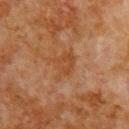Clinical impression: No biopsy was performed on this lesion — it was imaged during a full skin examination and was not determined to be concerning. Background: The lesion-visualizer software estimated a footprint of about 6 mm², an outline eccentricity of about 0.75 (0 = round, 1 = elongated), and a shape-asymmetry score of about 0.45 (0 = symmetric). The lesion is on the right upper arm. A 15 mm close-up extracted from a 3D total-body photography capture. Longest diameter approximately 4 mm. A male patient, roughly 80 years of age. Captured under cross-polarized illumination.